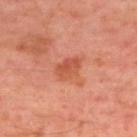Part of a total-body skin-imaging series; this lesion was reviewed on a skin check and was not flagged for biopsy.
The recorded lesion diameter is about 3.5 mm.
A region of skin cropped from a whole-body photographic capture, roughly 15 mm wide.
This is a cross-polarized tile.
The subject is a male in their 50s.
The total-body-photography lesion software estimated an area of roughly 6.5 mm², an eccentricity of roughly 0.75, and two-axis asymmetry of about 0.2. The software also gave a lesion color around L≈54 a*≈31 b*≈36 in CIELAB, about 9 CIELAB-L* units darker than the surrounding skin, and a lesion-to-skin contrast of about 6.5 (normalized; higher = more distinct).
From the upper back.